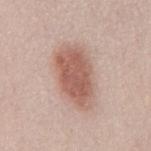Impression: The lesion was photographed on a routine skin check and not biopsied; there is no pathology result. Image and clinical context: A 15 mm close-up extracted from a 3D total-body photography capture. From the mid back. The patient is a female aged around 50. The lesion-visualizer software estimated an eccentricity of roughly 0.85 and two-axis asymmetry of about 0.15. The software also gave an automated nevus-likeness rating near 100 out of 100. About 7.5 mm across.